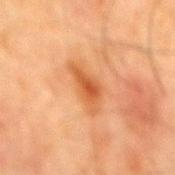Assessment:
No biopsy was performed on this lesion — it was imaged during a full skin examination and was not determined to be concerning.
Background:
Located on the mid back. A roughly 15 mm field-of-view crop from a total-body skin photograph. The patient is a male in their mid- to late 60s.